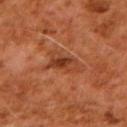Impression:
Recorded during total-body skin imaging; not selected for excision or biopsy.
Context:
A roughly 15 mm field-of-view crop from a total-body skin photograph. Captured under cross-polarized illumination. A male subject about 60 years old. Longest diameter approximately 4.5 mm. From the right upper arm.